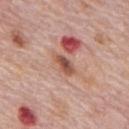follow-up=total-body-photography surveillance lesion; no biopsy.